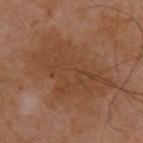This lesion was catalogued during total-body skin photography and was not selected for biopsy. A region of skin cropped from a whole-body photographic capture, roughly 15 mm wide. A male patient, about 40 years old. On the upper back.